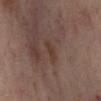Q: Who is the patient?
A: female, about 55 years old
Q: How was this image acquired?
A: ~15 mm tile from a whole-body skin photo
Q: Where on the body is the lesion?
A: the right lower leg
Q: How large is the lesion?
A: ≈2.5 mm